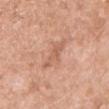| key | value |
|---|---|
| biopsy status | total-body-photography surveillance lesion; no biopsy |
| imaging modality | 15 mm crop, total-body photography |
| subject | female, about 55 years old |
| TBP lesion metrics | an eccentricity of roughly 0.95 and two-axis asymmetry of about 0.4; an automated nevus-likeness rating near 0 out of 100 and a detector confidence of about 100 out of 100 that the crop contains a lesion |
| location | the left upper arm |